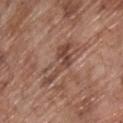<lesion>
<biopsy_status>not biopsied; imaged during a skin examination</biopsy_status>
<patient>
  <sex>male</sex>
  <age_approx>70</age_approx>
</patient>
<lesion_size>
  <long_diameter_mm_approx>6.5</long_diameter_mm_approx>
</lesion_size>
<site>upper back</site>
<image>
  <source>total-body photography crop</source>
  <field_of_view_mm>15</field_of_view_mm>
</image>
<lighting>white-light</lighting>
</lesion>